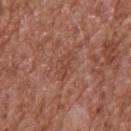Approximately 3.5 mm at its widest.
An algorithmic analysis of the crop reported a lesion area of about 5 mm² and two-axis asymmetry of about 0.35. The software also gave roughly 6 lightness units darker than nearby skin. The analysis additionally found a border-irregularity index near 4/10 and peripheral color asymmetry of about 1. And it measured a nevus-likeness score of about 0/100 and lesion-presence confidence of about 85/100.
A lesion tile, about 15 mm wide, cut from a 3D total-body photograph.
Imaged with white-light lighting.
Located on the upper back.
The subject is a male about 75 years old.